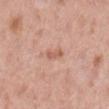Acquisition and patient details: The lesion is located on the right lower leg. This is a white-light tile. Cropped from a whole-body photographic skin survey; the tile spans about 15 mm. A female patient, aged 48–52.